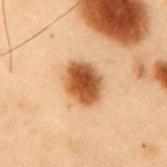workup: imaged on a skin check; not biopsied | patient: male, approximately 55 years of age | automated lesion analysis: an area of roughly 12 mm², a shape eccentricity near 0.65, and a shape-asymmetry score of about 0.15 (0 = symmetric); a border-irregularity rating of about 1.5/10, a within-lesion color-variation index near 5.5/10, and radial color variation of about 1.5; an automated nevus-likeness rating near 100 out of 100 and a detector confidence of about 100 out of 100 that the crop contains a lesion | tile lighting: cross-polarized illumination | imaging modality: ~15 mm crop, total-body skin-cancer survey | location: the mid back | lesion diameter: about 4.5 mm.